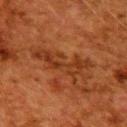Recorded during total-body skin imaging; not selected for excision or biopsy. The lesion is located on the upper back. Cropped from a total-body skin-imaging series; the visible field is about 15 mm. A female patient, approximately 50 years of age.